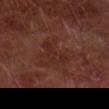biopsy status = no biopsy performed (imaged during a skin exam); anatomic site = the right forearm; image-analysis metrics = a shape eccentricity near 0.9 and a symmetry-axis asymmetry near 0.5; image source = 15 mm crop, total-body photography; tile lighting = cross-polarized illumination; size = ~4 mm (longest diameter); patient = male, roughly 65 years of age.